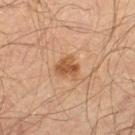A male subject about 60 years old. From the left thigh. A 15 mm close-up extracted from a 3D total-body photography capture. Captured under cross-polarized illumination. The lesion's longest dimension is about 2.5 mm.